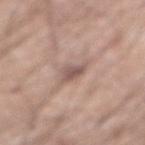biopsy status: imaged on a skin check; not biopsied
lighting: white-light
acquisition: 15 mm crop, total-body photography
body site: the abdomen
subject: male, aged 73–77